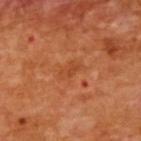{"biopsy_status": "not biopsied; imaged during a skin examination", "patient": {"sex": "female", "age_approx": 55}, "image": {"source": "total-body photography crop", "field_of_view_mm": 15}, "lighting": "cross-polarized", "site": "upper back", "lesion_size": {"long_diameter_mm_approx": 3.0}}